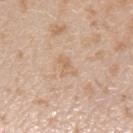Recorded during total-body skin imaging; not selected for excision or biopsy.